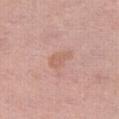Case summary:
– biopsy status — catalogued during a skin exam; not biopsied
– anatomic site — the right thigh
– image source — total-body-photography crop, ~15 mm field of view
– illumination — white-light
– diameter — ≈3 mm
– patient — female, aged around 55
– image-analysis metrics — an average lesion color of about L≈61 a*≈22 b*≈29 (CIELAB); border irregularity of about 3.5 on a 0–10 scale, a color-variation rating of about 1.5/10, and radial color variation of about 0.5; an automated nevus-likeness rating near 0 out of 100 and a lesion-detection confidence of about 100/100